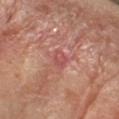* patient: female, in their mid- to late 50s
* imaging modality: ~15 mm crop, total-body skin-cancer survey
* tile lighting: cross-polarized illumination
* anatomic site: the left forearm
* size: ≈2.5 mm